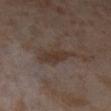Recorded during total-body skin imaging; not selected for excision or biopsy. A close-up tile cropped from a whole-body skin photograph, about 15 mm across. The patient is a female aged approximately 55. Located on the left lower leg. Measured at roughly 5 mm in maximum diameter. Captured under cross-polarized illumination.